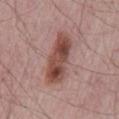The lesion was tiled from a total-body skin photograph and was not biopsied. Captured under white-light illumination. From the mid back. A 15 mm close-up extracted from a 3D total-body photography capture. Approximately 7 mm at its widest. An algorithmic analysis of the crop reported a lesion area of about 17 mm² and a shape-asymmetry score of about 0.2 (0 = symmetric). And it measured an automated nevus-likeness rating near 90 out of 100 and lesion-presence confidence of about 100/100. A male subject in their mid-60s.